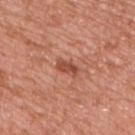patient = male, aged around 45 | tile lighting = white-light illumination | image source = 15 mm crop, total-body photography | diameter = ≈2.5 mm | site = the chest.